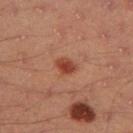Recorded during total-body skin imaging; not selected for excision or biopsy.
The tile uses cross-polarized illumination.
A male patient aged 43–47.
About 2.5 mm across.
The lesion is located on the left thigh.
A 15 mm close-up tile from a total-body photography series done for melanoma screening.